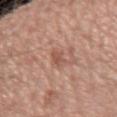Q: Was this lesion biopsied?
A: no biopsy performed (imaged during a skin exam)
Q: Where on the body is the lesion?
A: the right forearm
Q: Who is the patient?
A: female, aged 48–52
Q: What kind of image is this?
A: ~15 mm crop, total-body skin-cancer survey